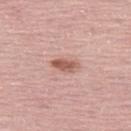Captured during whole-body skin photography for melanoma surveillance; the lesion was not biopsied.
On the left thigh.
Automated tile analysis of the lesion measured a mean CIELAB color near L≈57 a*≈23 b*≈27 and a lesion-to-skin contrast of about 8.5 (normalized; higher = more distinct). The software also gave a peripheral color-asymmetry measure near 0.5. The analysis additionally found a nevus-likeness score of about 95/100 and a lesion-detection confidence of about 100/100.
Measured at roughly 3 mm in maximum diameter.
A 15 mm close-up extracted from a 3D total-body photography capture.
Captured under white-light illumination.
A female patient, about 60 years old.